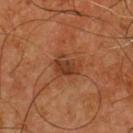Clinical impression: Recorded during total-body skin imaging; not selected for excision or biopsy. Background: Imaged with cross-polarized lighting. This image is a 15 mm lesion crop taken from a total-body photograph. Located on the chest. A male subject, aged 53–57. The recorded lesion diameter is about 3 mm. Automated tile analysis of the lesion measured a lesion area of about 6 mm² and an eccentricity of roughly 0.65. And it measured a mean CIELAB color near L≈35 a*≈23 b*≈31 and roughly 9 lightness units darker than nearby skin. It also reported border irregularity of about 4.5 on a 0–10 scale, a color-variation rating of about 2.5/10, and a peripheral color-asymmetry measure near 0.5.